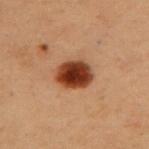anatomic site: the upper back | automated metrics: an area of roughly 11 mm², an outline eccentricity of about 0.65 (0 = round, 1 = elongated), and a shape-asymmetry score of about 0.15 (0 = symmetric); a border-irregularity rating of about 1.5/10, internal color variation of about 5.5 on a 0–10 scale, and a peripheral color-asymmetry measure near 1.5 | lighting: cross-polarized illumination | lesion diameter: about 4 mm | subject: female, in their 40s | image source: ~15 mm tile from a whole-body skin photo | diagnosis: a dysplastic (Clark) nevus.